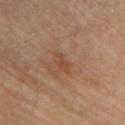workup: total-body-photography surveillance lesion; no biopsy
patient: male, aged around 85 or older
size: ~2.5 mm (longest diameter)
tile lighting: cross-polarized
imaging modality: ~15 mm crop, total-body skin-cancer survey
site: the back